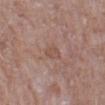No biopsy was performed on this lesion — it was imaged during a full skin examination and was not determined to be concerning. The subject is a male aged 78 to 82. On the leg. A 15 mm close-up extracted from a 3D total-body photography capture.